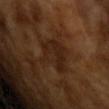Findings:
* notes · catalogued during a skin exam; not biopsied
* image · total-body-photography crop, ~15 mm field of view
* subject · male, in their mid-60s
* lesion size · about 5 mm